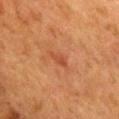{
  "biopsy_status": "not biopsied; imaged during a skin examination",
  "site": "mid back",
  "patient": {
    "sex": "female",
    "age_approx": 55
  },
  "image": {
    "source": "total-body photography crop",
    "field_of_view_mm": 15
  },
  "lesion_size": {
    "long_diameter_mm_approx": 3.0
  },
  "lighting": "cross-polarized"
}